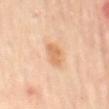anatomic site: the mid back
lighting: cross-polarized illumination
subject: female, aged approximately 60
diameter: ~3.5 mm (longest diameter)
imaging modality: ~15 mm tile from a whole-body skin photo